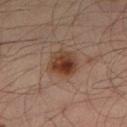Findings:
* biopsy status: catalogued during a skin exam; not biopsied
* body site: the leg
* diameter: ≈4 mm
* image source: 15 mm crop, total-body photography
* tile lighting: cross-polarized
* patient: male, aged approximately 35
* automated lesion analysis: a footprint of about 10 mm², a shape eccentricity near 0.55, and two-axis asymmetry of about 0.15; roughly 10 lightness units darker than nearby skin and a normalized border contrast of about 10; a color-variation rating of about 7.5/10 and radial color variation of about 2.5; an automated nevus-likeness rating near 95 out of 100 and lesion-presence confidence of about 100/100